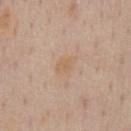Q: Was a biopsy performed?
A: imaged on a skin check; not biopsied
Q: What kind of image is this?
A: ~15 mm tile from a whole-body skin photo
Q: Patient demographics?
A: male, in their 60s
Q: How was the tile lit?
A: white-light
Q: Where on the body is the lesion?
A: the front of the torso
Q: Automated lesion metrics?
A: a detector confidence of about 100 out of 100 that the crop contains a lesion